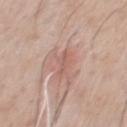Measured at roughly 2.5 mm in maximum diameter.
A male subject aged around 60.
On the chest.
A 15 mm crop from a total-body photograph taken for skin-cancer surveillance.
Imaged with white-light lighting.